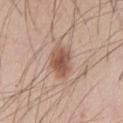A male subject in their mid-40s.
This image is a 15 mm lesion crop taken from a total-body photograph.
Measured at roughly 3.5 mm in maximum diameter.
Located on the chest.
The tile uses white-light illumination.